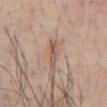Acquisition and patient details:
Imaged with white-light lighting. The recorded lesion diameter is about 2.5 mm. A male subject, about 55 years old. A 15 mm close-up tile from a total-body photography series done for melanoma screening. The lesion is on the front of the torso.